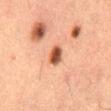notes: imaged on a skin check; not biopsied | image source: 15 mm crop, total-body photography | lesion size: ≈2.5 mm | illumination: cross-polarized | subject: male, aged approximately 50 | body site: the lower back.